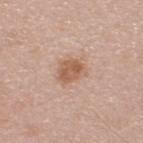A lesion tile, about 15 mm wide, cut from a 3D total-body photograph. A male subject aged approximately 45. The lesion-visualizer software estimated an automated nevus-likeness rating near 65 out of 100. Imaged with white-light lighting. Longest diameter approximately 3.5 mm. From the upper back.